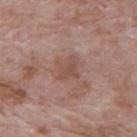| field | value |
|---|---|
| illumination | white-light illumination |
| lesion diameter | ≈3 mm |
| location | the mid back |
| automated metrics | a mean CIELAB color near L≈50 a*≈19 b*≈24, roughly 7 lightness units darker than nearby skin, and a lesion-to-skin contrast of about 5.5 (normalized; higher = more distinct); border irregularity of about 3 on a 0–10 scale and a color-variation rating of about 2/10; a nevus-likeness score of about 0/100 and a detector confidence of about 100 out of 100 that the crop contains a lesion |
| patient | male, aged approximately 60 |
| imaging modality | total-body-photography crop, ~15 mm field of view |